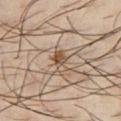workup = no biopsy performed (imaged during a skin exam).